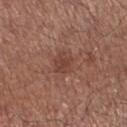This lesion was catalogued during total-body skin photography and was not selected for biopsy. The lesion is on the leg. A lesion tile, about 15 mm wide, cut from a 3D total-body photograph. The subject is a male roughly 70 years of age.